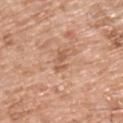| feature | finding |
|---|---|
| notes | total-body-photography surveillance lesion; no biopsy |
| diameter | ~3 mm (longest diameter) |
| patient | male, about 75 years old |
| image | ~15 mm crop, total-body skin-cancer survey |
| site | the upper back |
| tile lighting | white-light |